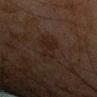Part of a total-body skin-imaging series; this lesion was reviewed on a skin check and was not flagged for biopsy. Imaged with cross-polarized lighting. Measured at roughly 5 mm in maximum diameter. From the right forearm. A close-up tile cropped from a whole-body skin photograph, about 15 mm across. An algorithmic analysis of the crop reported a lesion area of about 9 mm², an eccentricity of roughly 0.9, and a symmetry-axis asymmetry near 0.2. The software also gave an automated nevus-likeness rating near 0 out of 100 and lesion-presence confidence of about 100/100. A male patient about 45 years old.